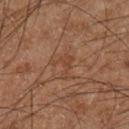Clinical impression: Imaged during a routine full-body skin examination; the lesion was not biopsied and no histopathology is available. Image and clinical context: The lesion's longest dimension is about 3 mm. A 15 mm close-up tile from a total-body photography series done for melanoma screening. The subject is a male in their 60s. On the left lower leg. The tile uses cross-polarized illumination. An algorithmic analysis of the crop reported a lesion color around L≈38 a*≈19 b*≈28 in CIELAB and a normalized border contrast of about 5. The software also gave a border-irregularity rating of about 8.5/10.